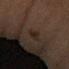Assessment: Captured during whole-body skin photography for melanoma surveillance; the lesion was not biopsied. Image and clinical context: A female subject, about 60 years old. An algorithmic analysis of the crop reported a lesion area of about 3 mm², an outline eccentricity of about 0.95 (0 = round, 1 = elongated), and two-axis asymmetry of about 0.2. The software also gave border irregularity of about 3 on a 0–10 scale and a color-variation rating of about 0/10. And it measured an automated nevus-likeness rating near 0 out of 100 and a lesion-detection confidence of about 90/100. A close-up tile cropped from a whole-body skin photograph, about 15 mm across. On the left forearm. This is a cross-polarized tile.